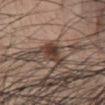Q: Was a biopsy performed?
A: catalogued during a skin exam; not biopsied
Q: How was this image acquired?
A: 15 mm crop, total-body photography
Q: Patient demographics?
A: male, in their mid- to late 50s
Q: Lesion size?
A: about 3.5 mm
Q: What lighting was used for the tile?
A: white-light illumination
Q: Where on the body is the lesion?
A: the abdomen
Q: Automated lesion metrics?
A: border irregularity of about 4.5 on a 0–10 scale, internal color variation of about 5.5 on a 0–10 scale, and a peripheral color-asymmetry measure near 2